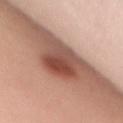<tbp_lesion>
<lesion_size>
  <long_diameter_mm_approx>5.5</long_diameter_mm_approx>
</lesion_size>
<site>back</site>
<patient>
  <sex>female</sex>
  <age_approx>40</age_approx>
</patient>
<image>
  <source>total-body photography crop</source>
  <field_of_view_mm>15</field_of_view_mm>
</image>
<lighting>white-light</lighting>
</tbp_lesion>